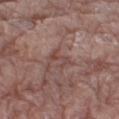The lesion is on the leg. Approximately 2.5 mm at its widest. The subject is a male roughly 80 years of age. The tile uses white-light illumination. A 15 mm close-up tile from a total-body photography series done for melanoma screening. The total-body-photography lesion software estimated a lesion area of about 3.5 mm², an eccentricity of roughly 0.7, and two-axis asymmetry of about 0.45.